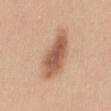Notes:
– workup: catalogued during a skin exam; not biopsied
– imaging modality: ~15 mm crop, total-body skin-cancer survey
– TBP lesion metrics: an area of roughly 17 mm², an outline eccentricity of about 0.9 (0 = round, 1 = elongated), and a symmetry-axis asymmetry near 0.2; an average lesion color of about L≈58 a*≈21 b*≈32 (CIELAB), a lesion–skin lightness drop of about 13, and a normalized border contrast of about 8.5; a border-irregularity rating of about 3/10; a nevus-likeness score of about 90/100 and a lesion-detection confidence of about 100/100
– anatomic site: the back
– size: ≈7 mm
– patient: female, aged approximately 45